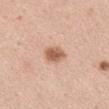Recorded during total-body skin imaging; not selected for excision or biopsy.
Automated tile analysis of the lesion measured a footprint of about 5 mm², a shape eccentricity near 0.65, and two-axis asymmetry of about 0.15.
The tile uses white-light illumination.
The recorded lesion diameter is about 3 mm.
On the mid back.
A 15 mm crop from a total-body photograph taken for skin-cancer surveillance.
The patient is a female aged around 40.